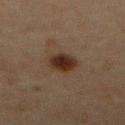Q: Was this lesion biopsied?
A: no biopsy performed (imaged during a skin exam)
Q: Patient demographics?
A: male, in their mid-70s
Q: Where on the body is the lesion?
A: the front of the torso
Q: Illumination type?
A: cross-polarized illumination
Q: How was this image acquired?
A: ~15 mm tile from a whole-body skin photo